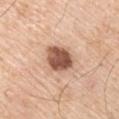Q: Was this lesion biopsied?
A: catalogued during a skin exam; not biopsied
Q: What is the imaging modality?
A: total-body-photography crop, ~15 mm field of view
Q: Patient demographics?
A: male, aged around 55
Q: Automated lesion metrics?
A: a footprint of about 11 mm², an eccentricity of roughly 0.65, and two-axis asymmetry of about 0.15; an automated nevus-likeness rating near 65 out of 100 and a detector confidence of about 100 out of 100 that the crop contains a lesion
Q: What is the lesion's diameter?
A: ≈4 mm
Q: What is the anatomic site?
A: the left upper arm
Q: Illumination type?
A: white-light